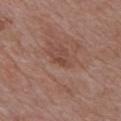Q: Was a biopsy performed?
A: catalogued during a skin exam; not biopsied
Q: Lesion size?
A: ≈1.5 mm
Q: What are the patient's age and sex?
A: male, about 70 years old
Q: What is the anatomic site?
A: the front of the torso
Q: What lighting was used for the tile?
A: white-light illumination
Q: What kind of image is this?
A: 15 mm crop, total-body photography